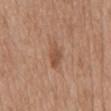Assessment: Captured during whole-body skin photography for melanoma surveillance; the lesion was not biopsied. Background: A female subject about 75 years old. The tile uses white-light illumination. The lesion is on the mid back. A lesion tile, about 15 mm wide, cut from a 3D total-body photograph.